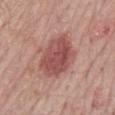Background: The recorded lesion diameter is about 5.5 mm. A male patient aged 68–72. From the back. The tile uses white-light illumination. This image is a 15 mm lesion crop taken from a total-body photograph. The total-body-photography lesion software estimated a footprint of about 18 mm² and a symmetry-axis asymmetry near 0.15. It also reported a border-irregularity index near 2/10 and radial color variation of about 1.5. And it measured an automated nevus-likeness rating near 90 out of 100 and lesion-presence confidence of about 100/100.